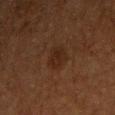Case summary:
- workup · catalogued during a skin exam; not biopsied
- image · 15 mm crop, total-body photography
- automated lesion analysis · a lesion color around L≈19 a*≈16 b*≈22 in CIELAB, roughly 5 lightness units darker than nearby skin, and a normalized border contrast of about 6.5; a border-irregularity index near 2/10
- lighting · cross-polarized
- subject · male, aged 63 to 67
- lesion size · about 2.5 mm
- body site · the chest